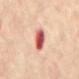biopsy_status: not biopsied; imaged during a skin examination
lesion_size:
  long_diameter_mm_approx: 4.0
lighting: cross-polarized
site: mid back
image:
  source: total-body photography crop
  field_of_view_mm: 15
patient:
  sex: male
  age_approx: 70